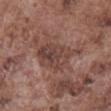Impression:
This lesion was catalogued during total-body skin photography and was not selected for biopsy.
Image and clinical context:
A close-up tile cropped from a whole-body skin photograph, about 15 mm across. From the abdomen. The tile uses white-light illumination. The patient is a male about 75 years old.